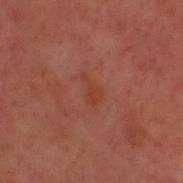follow-up — catalogued during a skin exam; not biopsied | subject — male, aged around 50 | location — the head or neck | acquisition — 15 mm crop, total-body photography.